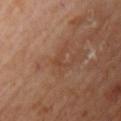| key | value |
|---|---|
| tile lighting | cross-polarized |
| image source | total-body-photography crop, ~15 mm field of view |
| patient | male, roughly 70 years of age |
| body site | the chest |
| size | ≈2.5 mm |